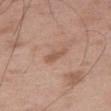Clinical impression:
Captured during whole-body skin photography for melanoma surveillance; the lesion was not biopsied.
Clinical summary:
The total-body-photography lesion software estimated an eccentricity of roughly 0.9 and a shape-asymmetry score of about 0.3 (0 = symmetric). The software also gave a lesion color around L≈55 a*≈20 b*≈29 in CIELAB and a normalized border contrast of about 6. The lesion is on the right thigh. This is a white-light tile. A region of skin cropped from a whole-body photographic capture, roughly 15 mm wide. A male subject aged 48–52. The lesion's longest dimension is about 3 mm.